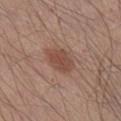Impression: Part of a total-body skin-imaging series; this lesion was reviewed on a skin check and was not flagged for biopsy. Background: Approximately 4 mm at its widest. The lesion is located on the right lower leg. This is a white-light tile. A male patient, aged 43–47. Cropped from a whole-body photographic skin survey; the tile spans about 15 mm.